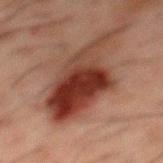Part of a total-body skin-imaging series; this lesion was reviewed on a skin check and was not flagged for biopsy. About 11.5 mm across. Located on the mid back. Imaged with cross-polarized lighting. A 15 mm close-up extracted from a 3D total-body photography capture. A male patient, approximately 50 years of age.